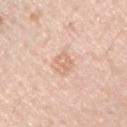No biopsy was performed on this lesion — it was imaged during a full skin examination and was not determined to be concerning. About 3 mm across. On the right upper arm. A male patient in their 60s. A 15 mm crop from a total-body photograph taken for skin-cancer surveillance. An algorithmic analysis of the crop reported a lesion area of about 5 mm², an outline eccentricity of about 0.8 (0 = round, 1 = elongated), and a shape-asymmetry score of about 0.2 (0 = symmetric). It also reported a border-irregularity rating of about 2.5/10, a within-lesion color-variation index near 3/10, and peripheral color asymmetry of about 1.5.